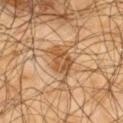The lesion was tiled from a total-body skin photograph and was not biopsied. The total-body-photography lesion software estimated an automated nevus-likeness rating near 50 out of 100 and lesion-presence confidence of about 100/100. The patient is a male aged 63–67. On the upper back. Imaged with cross-polarized lighting. A lesion tile, about 15 mm wide, cut from a 3D total-body photograph.